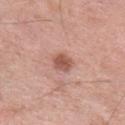<tbp_lesion>
<patient>
  <sex>male</sex>
  <age_approx>80</age_approx>
</patient>
<image>
  <source>total-body photography crop</source>
  <field_of_view_mm>15</field_of_view_mm>
</image>
<site>right lower leg</site>
</tbp_lesion>